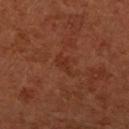Q: Was a biopsy performed?
A: catalogued during a skin exam; not biopsied
Q: Patient demographics?
A: female, aged approximately 65
Q: What lighting was used for the tile?
A: cross-polarized illumination
Q: How was this image acquired?
A: ~15 mm tile from a whole-body skin photo
Q: How large is the lesion?
A: ~2.5 mm (longest diameter)
Q: What is the anatomic site?
A: the left forearm